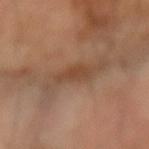Assessment: The lesion was tiled from a total-body skin photograph and was not biopsied. Image and clinical context: A 15 mm close-up extracted from a 3D total-body photography capture. About 3.5 mm across. A male patient aged 63 to 67. Located on the right forearm.